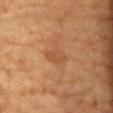The lesion was tiled from a total-body skin photograph and was not biopsied. The subject is a male aged 83 or over. From the chest. A 15 mm close-up tile from a total-body photography series done for melanoma screening.